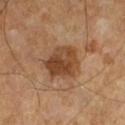Impression:
Recorded during total-body skin imaging; not selected for excision or biopsy.
Image and clinical context:
On the left lower leg. A close-up tile cropped from a whole-body skin photograph, about 15 mm across. A male patient, aged 63–67. Automated tile analysis of the lesion measured a lesion–skin lightness drop of about 11 and a normalized lesion–skin contrast near 9. The analysis additionally found an automated nevus-likeness rating near 50 out of 100 and a lesion-detection confidence of about 100/100. Approximately 4.5 mm at its widest. Imaged with cross-polarized lighting.